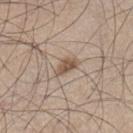A 15 mm close-up tile from a total-body photography series done for melanoma screening. The lesion-visualizer software estimated an average lesion color of about L≈53 a*≈15 b*≈28 (CIELAB) and a normalized border contrast of about 8. And it measured a color-variation rating of about 2/10 and a peripheral color-asymmetry measure near 1. The analysis additionally found a classifier nevus-likeness of about 85/100 and lesion-presence confidence of about 100/100. Longest diameter approximately 3 mm. A male patient about 45 years old. Imaged with white-light lighting. The lesion is located on the left lower leg.